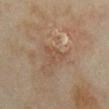Assessment:
The lesion was tiled from a total-body skin photograph and was not biopsied.